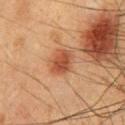The lesion was photographed on a routine skin check and not biopsied; there is no pathology result. The tile uses cross-polarized illumination. The lesion's longest dimension is about 3 mm. From the chest. A region of skin cropped from a whole-body photographic capture, roughly 15 mm wide. An algorithmic analysis of the crop reported internal color variation of about 3 on a 0–10 scale and a peripheral color-asymmetry measure near 1. The software also gave a classifier nevus-likeness of about 100/100 and a lesion-detection confidence of about 100/100. A male patient about 50 years old.